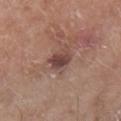No biopsy was performed on this lesion — it was imaged during a full skin examination and was not determined to be concerning. Cropped from a whole-body photographic skin survey; the tile spans about 15 mm. The total-body-photography lesion software estimated an area of roughly 5.5 mm² and an outline eccentricity of about 0.65 (0 = round, 1 = elongated). And it measured a lesion color around L≈44 a*≈20 b*≈22 in CIELAB and about 11 CIELAB-L* units darker than the surrounding skin. The lesion is located on the right lower leg. Captured under white-light illumination. A male subject, about 80 years old.